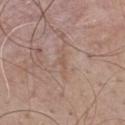| key | value |
|---|---|
| workup | total-body-photography surveillance lesion; no biopsy |
| site | the upper back |
| illumination | white-light |
| acquisition | total-body-photography crop, ~15 mm field of view |
| subject | male, aged approximately 45 |
| automated metrics | a lesion area of about 1 mm² and a symmetry-axis asymmetry near 0.4; a border-irregularity rating of about 3/10, a within-lesion color-variation index near 0/10, and peripheral color asymmetry of about 0; a nevus-likeness score of about 0/100 and lesion-presence confidence of about 90/100 |